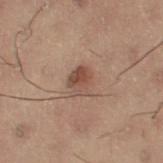The tile uses cross-polarized illumination.
From the leg.
Measured at roughly 3.5 mm in maximum diameter.
An algorithmic analysis of the crop reported an average lesion color of about L≈40 a*≈15 b*≈22 (CIELAB), roughly 8 lightness units darker than nearby skin, and a normalized lesion–skin contrast near 6.5. The software also gave a border-irregularity rating of about 1.5/10, internal color variation of about 5.5 on a 0–10 scale, and a peripheral color-asymmetry measure near 2.
The patient is a female aged approximately 55.
A lesion tile, about 15 mm wide, cut from a 3D total-body photograph.